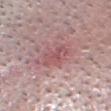  lesion_size:
    long_diameter_mm_approx: 3.5
  patient:
    sex: male
    age_approx: 50
  lighting: white-light
  automated_metrics:
    border_irregularity_0_10: 6.5
    color_variation_0_10: 2.5
    peripheral_color_asymmetry: 1.0
  image:
    source: total-body photography crop
    field_of_view_mm: 15
  site: head or neck